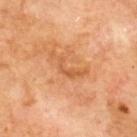Findings:
* notes · total-body-photography surveillance lesion; no biopsy
* diameter · ≈5 mm
* tile lighting · cross-polarized
* image · total-body-photography crop, ~15 mm field of view
* patient · male, roughly 70 years of age
* anatomic site · the upper back
* TBP lesion metrics · an outline eccentricity of about 0.9 (0 = round, 1 = elongated) and a symmetry-axis asymmetry near 0.75; an average lesion color of about L≈58 a*≈26 b*≈41 (CIELAB) and a lesion-to-skin contrast of about 5.5 (normalized; higher = more distinct); border irregularity of about 9.5 on a 0–10 scale, internal color variation of about 1 on a 0–10 scale, and a peripheral color-asymmetry measure near 0.5